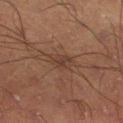The lesion was photographed on a routine skin check and not biopsied; there is no pathology result. Cropped from a whole-body photographic skin survey; the tile spans about 15 mm. The patient is a male about 55 years old. The total-body-photography lesion software estimated a footprint of about 5 mm². And it measured about 5 CIELAB-L* units darker than the surrounding skin and a normalized lesion–skin contrast near 5.5. And it measured a border-irregularity rating of about 2.5/10, a color-variation rating of about 3/10, and peripheral color asymmetry of about 1. Located on the leg.